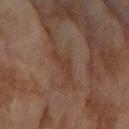anatomic site = the right forearm | lesion size = ~4 mm (longest diameter) | image = ~15 mm tile from a whole-body skin photo | lighting = cross-polarized illumination | image-analysis metrics = a lesion color around L≈31 a*≈16 b*≈24 in CIELAB, a lesion–skin lightness drop of about 5, and a normalized border contrast of about 6; border irregularity of about 6.5 on a 0–10 scale, a within-lesion color-variation index near 1/10, and a peripheral color-asymmetry measure near 0.5 | patient = female, roughly 80 years of age.